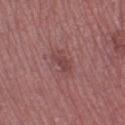follow-up=total-body-photography surveillance lesion; no biopsy
patient=female, about 45 years old
acquisition=~15 mm crop, total-body skin-cancer survey
automated lesion analysis=a border-irregularity rating of about 3.5/10; a nevus-likeness score of about 0/100 and a lesion-detection confidence of about 100/100
illumination=white-light
anatomic site=the left thigh